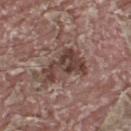On the upper back. A male subject, approximately 40 years of age. A region of skin cropped from a whole-body photographic capture, roughly 15 mm wide.